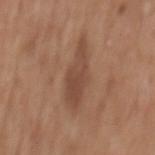– notes — total-body-photography surveillance lesion; no biopsy
– patient — male, in their 60s
– TBP lesion metrics — an area of roughly 10 mm², an eccentricity of roughly 0.9, and two-axis asymmetry of about 0.3; an automated nevus-likeness rating near 10 out of 100 and a detector confidence of about 100 out of 100 that the crop contains a lesion
– illumination — white-light illumination
– body site — the mid back
– size — about 6 mm
– image — total-body-photography crop, ~15 mm field of view